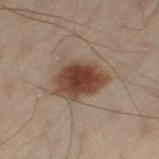Acquisition and patient details: Approximately 5.5 mm at its widest. This is a cross-polarized tile. On the leg. A 15 mm close-up extracted from a 3D total-body photography capture. The patient is a male aged approximately 50. The lesion-visualizer software estimated an area of roughly 15 mm² and a symmetry-axis asymmetry near 0.2. And it measured a mean CIELAB color near L≈33 a*≈15 b*≈23, about 12 CIELAB-L* units darker than the surrounding skin, and a lesion-to-skin contrast of about 11.5 (normalized; higher = more distinct). The software also gave border irregularity of about 2 on a 0–10 scale, a within-lesion color-variation index near 4/10, and peripheral color asymmetry of about 1.